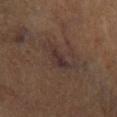biopsy status = total-body-photography surveillance lesion; no biopsy | image source = ~15 mm tile from a whole-body skin photo | diameter = ~4 mm (longest diameter) | site = the left lower leg | tile lighting = cross-polarized illumination | patient = male, aged 58–62.